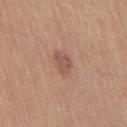Assessment: Part of a total-body skin-imaging series; this lesion was reviewed on a skin check and was not flagged for biopsy. Acquisition and patient details: The tile uses white-light illumination. The total-body-photography lesion software estimated an eccentricity of roughly 0.75. The analysis additionally found a lesion color around L≈53 a*≈23 b*≈27 in CIELAB, a lesion–skin lightness drop of about 9, and a normalized border contrast of about 6.5. The recorded lesion diameter is about 3 mm. A roughly 15 mm field-of-view crop from a total-body skin photograph. The patient is a female aged around 65. The lesion is on the right thigh.